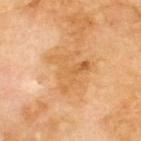Assessment:
Captured during whole-body skin photography for melanoma surveillance; the lesion was not biopsied.
Background:
A male subject aged 68 to 72. Automated tile analysis of the lesion measured a lesion color around L≈61 a*≈23 b*≈43 in CIELAB and a normalized lesion–skin contrast near 5.5. From the upper back. Cropped from a total-body skin-imaging series; the visible field is about 15 mm.